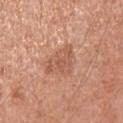Case summary:
- biopsy status — catalogued during a skin exam; not biopsied
- tile lighting — white-light
- body site — the right upper arm
- diameter — about 4 mm
- image source — 15 mm crop, total-body photography
- subject — male, aged 53 to 57